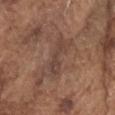Acquisition and patient details:
This is a white-light tile. Cropped from a whole-body photographic skin survey; the tile spans about 15 mm. Measured at roughly 4 mm in maximum diameter. The lesion is on the head or neck. A male subject, aged 73 to 77. The total-body-photography lesion software estimated a footprint of about 6 mm² and an outline eccentricity of about 0.9 (0 = round, 1 = elongated). The software also gave a normalized border contrast of about 5.5.